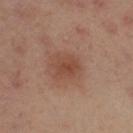{"biopsy_status": "not biopsied; imaged during a skin examination", "lighting": "cross-polarized", "image": {"source": "total-body photography crop", "field_of_view_mm": 15}, "patient": {"sex": "female", "age_approx": 40}, "lesion_size": {"long_diameter_mm_approx": 3.0}, "site": "right upper arm"}